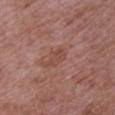Recorded during total-body skin imaging; not selected for excision or biopsy. A female patient, aged 68–72. From the right upper arm. A region of skin cropped from a whole-body photographic capture, roughly 15 mm wide.